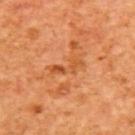Clinical impression: The lesion was photographed on a routine skin check and not biopsied; there is no pathology result. Acquisition and patient details: A 15 mm close-up tile from a total-body photography series done for melanoma screening. Captured under cross-polarized illumination. Measured at roughly 4 mm in maximum diameter. The patient is a female aged around 40. Located on the upper back.